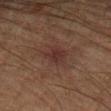Notes:
* lighting · cross-polarized
* location · the left upper arm
* imaging modality · ~15 mm tile from a whole-body skin photo
* lesion size · ≈3 mm
* subject · male, aged approximately 65